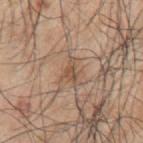follow-up: catalogued during a skin exam; not biopsied | patient: male, in their 70s | site: the left upper arm | illumination: white-light | acquisition: 15 mm crop, total-body photography.